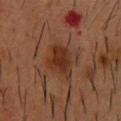Clinical impression: The lesion was tiled from a total-body skin photograph and was not biopsied. Clinical summary: Automated image analysis of the tile measured a lesion area of about 11 mm², an eccentricity of roughly 0.75, and two-axis asymmetry of about 0.25. And it measured a lesion color around L≈27 a*≈19 b*≈26 in CIELAB and a lesion–skin lightness drop of about 8. Imaged with cross-polarized lighting. From the chest. A male patient, aged 53 to 57. A 15 mm crop from a total-body photograph taken for skin-cancer surveillance. Longest diameter approximately 5 mm.